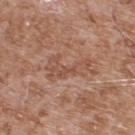Case summary:
• follow-up — no biopsy performed (imaged during a skin exam)
• tile lighting — white-light illumination
• image source — total-body-photography crop, ~15 mm field of view
• body site — the upper back
• patient — male, roughly 55 years of age